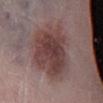Q: What is the lesion's diameter?
A: ≈7.5 mm
Q: Where on the body is the lesion?
A: the leg
Q: Automated lesion metrics?
A: a border-irregularity index near 2.5/10, a within-lesion color-variation index near 5/10, and a peripheral color-asymmetry measure near 1.5
Q: How was this image acquired?
A: ~15 mm crop, total-body skin-cancer survey
Q: Patient demographics?
A: male, about 55 years old
Q: Illumination type?
A: white-light